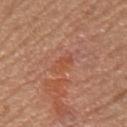Impression:
No biopsy was performed on this lesion — it was imaged during a full skin examination and was not determined to be concerning.
Image and clinical context:
A 15 mm close-up tile from a total-body photography series done for melanoma screening. The lesion is on the right forearm. Longest diameter approximately 3.5 mm. Imaged with white-light lighting. A female subject aged 58 to 62.